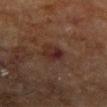Assessment: Part of a total-body skin-imaging series; this lesion was reviewed on a skin check and was not flagged for biopsy. Acquisition and patient details: Approximately 3 mm at its widest. A region of skin cropped from a whole-body photographic capture, roughly 15 mm wide. A female patient roughly 80 years of age. The lesion is on the right forearm. This is a cross-polarized tile.